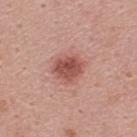{
  "biopsy_status": "not biopsied; imaged during a skin examination",
  "site": "upper back",
  "automated_metrics": {
    "cielab_L": 51,
    "cielab_a": 27,
    "cielab_b": 26,
    "vs_skin_darker_L": 13.0,
    "vs_skin_contrast_norm": 8.5,
    "border_irregularity_0_10": 2.0,
    "peripheral_color_asymmetry": 1.0,
    "nevus_likeness_0_100": 95
  },
  "patient": {
    "sex": "male",
    "age_approx": 45
  },
  "image": {
    "source": "total-body photography crop",
    "field_of_view_mm": 15
  },
  "lesion_size": {
    "long_diameter_mm_approx": 3.5
  }
}